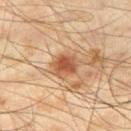Case summary:
- automated metrics · a shape eccentricity near 0.6 and a shape-asymmetry score of about 0.15 (0 = symmetric); an average lesion color of about L≈45 a*≈19 b*≈30 (CIELAB), roughly 10 lightness units darker than nearby skin, and a normalized border contrast of about 8
- diameter · about 4 mm
- subject · male, aged around 45
- acquisition · total-body-photography crop, ~15 mm field of view
- anatomic site · the right thigh